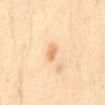{"biopsy_status": "not biopsied; imaged during a skin examination", "patient": {"sex": "male", "age_approx": 45}, "lighting": "cross-polarized", "site": "front of the torso", "image": {"source": "total-body photography crop", "field_of_view_mm": 15}, "automated_metrics": {"area_mm2_approx": 3.0, "eccentricity": 0.75, "shape_asymmetry": 0.3, "cielab_L": 73, "cielab_a": 18, "cielab_b": 42, "vs_skin_darker_L": 10.0, "border_irregularity_0_10": 2.5, "color_variation_0_10": 2.0, "peripheral_color_asymmetry": 1.0, "nevus_likeness_0_100": 10, "lesion_detection_confidence_0_100": 100}}